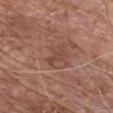biopsy_status: not biopsied; imaged during a skin examination
automated_metrics:
  eccentricity: 0.95
  shape_asymmetry: 0.4
  cielab_L: 45
  cielab_a: 22
  cielab_b: 27
  vs_skin_contrast_norm: 5.5
site: chest
lesion_size:
  long_diameter_mm_approx: 3.5
patient:
  sex: male
  age_approx: 65
image:
  source: total-body photography crop
  field_of_view_mm: 15